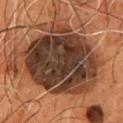workup: catalogued during a skin exam; not biopsied
automated metrics: an area of roughly 60 mm², an eccentricity of roughly 0.65, and a shape-asymmetry score of about 0.15 (0 = symmetric); about 17 CIELAB-L* units darker than the surrounding skin; a within-lesion color-variation index near 8/10 and peripheral color asymmetry of about 2.5
lesion size: about 11 mm
illumination: cross-polarized illumination
subject: male, aged 53 to 57
site: the front of the torso
image: total-body-photography crop, ~15 mm field of view